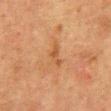Recorded during total-body skin imaging; not selected for excision or biopsy. An algorithmic analysis of the crop reported a footprint of about 3 mm² and an outline eccentricity of about 0.9 (0 = round, 1 = elongated). The software also gave a lesion color around L≈44 a*≈21 b*≈34 in CIELAB, roughly 7 lightness units darker than nearby skin, and a normalized lesion–skin contrast near 6. It also reported a border-irregularity rating of about 5/10, internal color variation of about 0.5 on a 0–10 scale, and a peripheral color-asymmetry measure near 0. And it measured an automated nevus-likeness rating near 0 out of 100. The patient is a female approximately 50 years of age. Cropped from a total-body skin-imaging series; the visible field is about 15 mm. The tile uses cross-polarized illumination. The lesion is located on the abdomen.